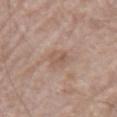This lesion was catalogued during total-body skin photography and was not selected for biopsy. A male subject about 70 years old. Located on the left upper arm. About 2.5 mm across. This image is a 15 mm lesion crop taken from a total-body photograph. Captured under white-light illumination. The lesion-visualizer software estimated an area of roughly 4 mm², an outline eccentricity of about 0.75 (0 = round, 1 = elongated), and a shape-asymmetry score of about 0.25 (0 = symmetric). And it measured an automated nevus-likeness rating near 0 out of 100 and a lesion-detection confidence of about 100/100.